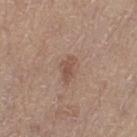biopsy status: total-body-photography surveillance lesion; no biopsy | diameter: ≈3 mm | patient: male, in their mid- to late 60s | location: the left thigh | TBP lesion metrics: a lesion color around L≈51 a*≈19 b*≈26 in CIELAB, a lesion–skin lightness drop of about 8, and a lesion-to-skin contrast of about 6.5 (normalized; higher = more distinct) | image source: 15 mm crop, total-body photography.